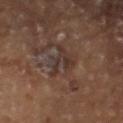<record>
<biopsy_status>not biopsied; imaged during a skin examination</biopsy_status>
<lesion_size>
  <long_diameter_mm_approx>5.0</long_diameter_mm_approx>
</lesion_size>
<image>
  <source>total-body photography crop</source>
  <field_of_view_mm>15</field_of_view_mm>
</image>
<site>left forearm</site>
<lighting>cross-polarized</lighting>
<patient>
  <sex>female</sex>
  <age_approx>70</age_approx>
</patient>
<automated_metrics>
  <cielab_L>31</cielab_L>
  <cielab_a>14</cielab_a>
  <cielab_b>19</cielab_b>
  <vs_skin_darker_L>7.0</vs_skin_darker_L>
  <vs_skin_contrast_norm>7.0</vs_skin_contrast_norm>
</automated_metrics>
</record>